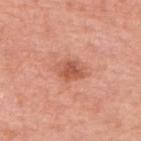Case summary:
* workup · no biopsy performed (imaged during a skin exam)
* body site · the upper back
* imaging modality · total-body-photography crop, ~15 mm field of view
* subject · female, aged approximately 75